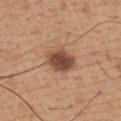<tbp_lesion>
  <biopsy_status>not biopsied; imaged during a skin examination</biopsy_status>
  <site>chest</site>
  <patient>
    <sex>male</sex>
    <age_approx>65</age_approx>
  </patient>
  <image>
    <source>total-body photography crop</source>
    <field_of_view_mm>15</field_of_view_mm>
  </image>
</tbp_lesion>